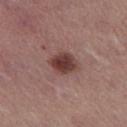Impression: Recorded during total-body skin imaging; not selected for excision or biopsy. Context: Longest diameter approximately 3 mm. A roughly 15 mm field-of-view crop from a total-body skin photograph. The lesion-visualizer software estimated a footprint of about 7.5 mm² and an outline eccentricity of about 0.55 (0 = round, 1 = elongated). The analysis additionally found an average lesion color of about L≈39 a*≈21 b*≈21 (CIELAB), a lesion–skin lightness drop of about 13, and a normalized border contrast of about 10.5. The software also gave a border-irregularity rating of about 1/10, internal color variation of about 4 on a 0–10 scale, and peripheral color asymmetry of about 1. The tile uses white-light illumination. A female patient, in their mid-50s. On the leg.